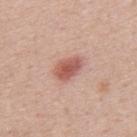Captured during whole-body skin photography for melanoma surveillance; the lesion was not biopsied.
Cropped from a whole-body photographic skin survey; the tile spans about 15 mm.
Captured under white-light illumination.
Longest diameter approximately 3.5 mm.
From the back.
Automated tile analysis of the lesion measured a classifier nevus-likeness of about 95/100.
A male patient about 40 years old.